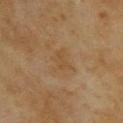Part of a total-body skin-imaging series; this lesion was reviewed on a skin check and was not flagged for biopsy.
A close-up tile cropped from a whole-body skin photograph, about 15 mm across.
On the upper back.
Approximately 3 mm at its widest.
An algorithmic analysis of the crop reported an area of roughly 5 mm², an eccentricity of roughly 0.7, and a symmetry-axis asymmetry near 0.35. The software also gave an average lesion color of about L≈44 a*≈15 b*≈33 (CIELAB), a lesion–skin lightness drop of about 5, and a normalized border contrast of about 5. The analysis additionally found a border-irregularity rating of about 4/10, internal color variation of about 1.5 on a 0–10 scale, and a peripheral color-asymmetry measure near 0.5. It also reported an automated nevus-likeness rating near 0 out of 100 and a lesion-detection confidence of about 100/100.
The tile uses cross-polarized illumination.
A female patient, aged 58–62.